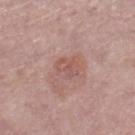Assessment: Recorded during total-body skin imaging; not selected for excision or biopsy. Clinical summary: A female subject, aged 58 to 62. Located on the right lower leg. Captured under white-light illumination. The lesion's longest dimension is about 2.5 mm. Cropped from a whole-body photographic skin survey; the tile spans about 15 mm.